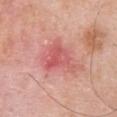A male subject, aged 53–57. The lesion's longest dimension is about 4.5 mm. A roughly 15 mm field-of-view crop from a total-body skin photograph. The lesion is on the chest. The lesion-visualizer software estimated a lesion color around L≈58 a*≈34 b*≈26 in CIELAB, roughly 10 lightness units darker than nearby skin, and a normalized border contrast of about 6.